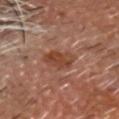Automated image analysis of the tile measured a classifier nevus-likeness of about 35/100.
A male patient, roughly 60 years of age.
From the head or neck.
A lesion tile, about 15 mm wide, cut from a 3D total-body photograph.
Approximately 4 mm at its widest.